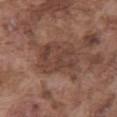This lesion was catalogued during total-body skin photography and was not selected for biopsy. The recorded lesion diameter is about 6 mm. A close-up tile cropped from a whole-body skin photograph, about 15 mm across. A male subject approximately 75 years of age. From the abdomen.